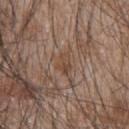Q: Was this lesion biopsied?
A: no biopsy performed (imaged during a skin exam)
Q: Lesion size?
A: ≈2.5 mm
Q: What kind of image is this?
A: ~15 mm tile from a whole-body skin photo
Q: Patient demographics?
A: male, in their mid-40s
Q: What lighting was used for the tile?
A: white-light illumination
Q: What is the anatomic site?
A: the upper back